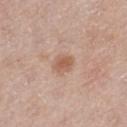Q: Was a biopsy performed?
A: no biopsy performed (imaged during a skin exam)
Q: How was this image acquired?
A: ~15 mm crop, total-body skin-cancer survey
Q: Who is the patient?
A: female, aged 58 to 62
Q: Lesion location?
A: the left thigh
Q: What lighting was used for the tile?
A: white-light
Q: What is the lesion's diameter?
A: ≈2.5 mm
Q: Automated lesion metrics?
A: a lesion color around L≈58 a*≈21 b*≈29 in CIELAB, about 9 CIELAB-L* units darker than the surrounding skin, and a lesion-to-skin contrast of about 7 (normalized; higher = more distinct); a border-irregularity index near 1.5/10, internal color variation of about 2.5 on a 0–10 scale, and peripheral color asymmetry of about 1; a nevus-likeness score of about 65/100